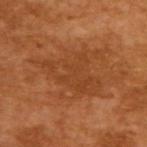Q: Was this lesion biopsied?
A: total-body-photography surveillance lesion; no biopsy
Q: How was this image acquired?
A: 15 mm crop, total-body photography
Q: What did automated image analysis measure?
A: an automated nevus-likeness rating near 0 out of 100 and a detector confidence of about 100 out of 100 that the crop contains a lesion
Q: Who is the patient?
A: male, aged approximately 65
Q: What lighting was used for the tile?
A: cross-polarized
Q: How large is the lesion?
A: ≈8 mm